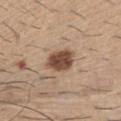| field | value |
|---|---|
| image | ~15 mm tile from a whole-body skin photo |
| body site | the abdomen |
| tile lighting | white-light |
| lesion size | about 3.5 mm |
| subject | male, aged 58–62 |